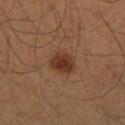Clinical impression:
The lesion was tiled from a total-body skin photograph and was not biopsied.
Image and clinical context:
Cropped from a total-body skin-imaging series; the visible field is about 15 mm. A male patient aged approximately 40. The lesion-visualizer software estimated a footprint of about 7 mm². And it measured a lesion-detection confidence of about 100/100. The lesion is on the leg. The lesion's longest dimension is about 3.5 mm. Captured under cross-polarized illumination.